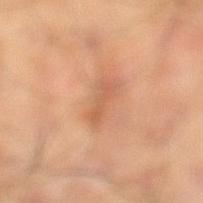Q: Was a biopsy performed?
A: no biopsy performed (imaged during a skin exam)
Q: What did automated image analysis measure?
A: a lesion area of about 3 mm², an outline eccentricity of about 0.95 (0 = round, 1 = elongated), and a shape-asymmetry score of about 0.45 (0 = symmetric); a mean CIELAB color near L≈55 a*≈23 b*≈34
Q: Who is the patient?
A: male, in their mid-60s
Q: What is the imaging modality?
A: total-body-photography crop, ~15 mm field of view
Q: What is the anatomic site?
A: the left lower leg
Q: How was the tile lit?
A: cross-polarized illumination
Q: Lesion size?
A: ~3 mm (longest diameter)